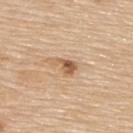Notes:
* image — ~15 mm tile from a whole-body skin photo
* image-analysis metrics — a classifier nevus-likeness of about 75/100
* subject — male, aged around 80
* illumination — white-light illumination
* lesion size — about 3 mm
* anatomic site — the upper back